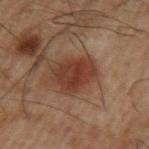Q: Was this lesion biopsied?
A: imaged on a skin check; not biopsied
Q: Patient demographics?
A: male, approximately 50 years of age
Q: What is the imaging modality?
A: 15 mm crop, total-body photography
Q: Lesion location?
A: the left upper arm